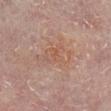The lesion was photographed on a routine skin check and not biopsied; there is no pathology result. The recorded lesion diameter is about 5.5 mm. A male subject, approximately 60 years of age. A roughly 15 mm field-of-view crop from a total-body skin photograph. The tile uses cross-polarized illumination. On the leg. The lesion-visualizer software estimated a footprint of about 11 mm², a shape eccentricity near 0.85, and a symmetry-axis asymmetry near 0.4. It also reported an automated nevus-likeness rating near 0 out of 100 and lesion-presence confidence of about 100/100.